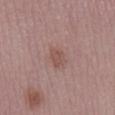follow-up: no biopsy performed (imaged during a skin exam)
acquisition: ~15 mm tile from a whole-body skin photo
subject: male, about 50 years old
location: the leg
illumination: white-light illumination